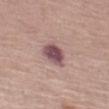* notes — catalogued during a skin exam; not biopsied
* patient — female, aged approximately 65
* body site — the left thigh
* acquisition — 15 mm crop, total-body photography